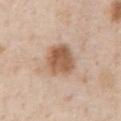| field | value |
|---|---|
| notes | total-body-photography surveillance lesion; no biopsy |
| body site | the chest |
| automated metrics | a lesion area of about 13 mm² and a shape-asymmetry score of about 0.15 (0 = symmetric); an average lesion color of about L≈58 a*≈19 b*≈32 (CIELAB); a border-irregularity index near 1.5/10, a color-variation rating of about 4.5/10, and radial color variation of about 1.5 |
| acquisition | ~15 mm tile from a whole-body skin photo |
| patient | male, roughly 50 years of age |